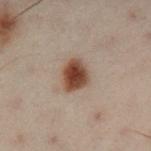patient: male, approximately 50 years of age
lighting: cross-polarized illumination
lesion diameter: ≈3.5 mm
acquisition: total-body-photography crop, ~15 mm field of view
site: the left lower leg
image-analysis metrics: a mean CIELAB color near L≈36 a*≈16 b*≈23, a lesion–skin lightness drop of about 14, and a normalized lesion–skin contrast near 12; a border-irregularity rating of about 1.5/10, internal color variation of about 5 on a 0–10 scale, and peripheral color asymmetry of about 1; an automated nevus-likeness rating near 100 out of 100 and a lesion-detection confidence of about 100/100